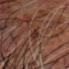Imaged during a routine full-body skin examination; the lesion was not biopsied and no histopathology is available. A 15 mm close-up tile from a total-body photography series done for melanoma screening. On the head or neck. A male patient approximately 65 years of age. The recorded lesion diameter is about 2.5 mm. This is a cross-polarized tile.